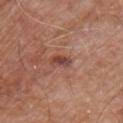{
  "biopsy_status": "not biopsied; imaged during a skin examination",
  "image": {
    "source": "total-body photography crop",
    "field_of_view_mm": 15
  },
  "site": "chest",
  "lesion_size": {
    "long_diameter_mm_approx": 2.5
  },
  "patient": {
    "sex": "male",
    "age_approx": 80
  },
  "lighting": "white-light"
}